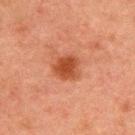notes = no biopsy performed (imaged during a skin exam).